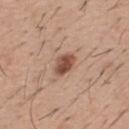biopsy status = catalogued during a skin exam; not biopsied | body site = the mid back | tile lighting = white-light | image = ~15 mm crop, total-body skin-cancer survey | patient = male, aged 38–42.